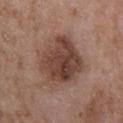Notes:
• notes — no biopsy performed (imaged during a skin exam)
• image source — ~15 mm tile from a whole-body skin photo
• anatomic site — the upper back
• subject — male, aged approximately 50